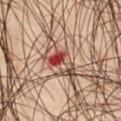Part of a total-body skin-imaging series; this lesion was reviewed on a skin check and was not flagged for biopsy. This image is a 15 mm lesion crop taken from a total-body photograph. From the right thigh. This is a cross-polarized tile. An algorithmic analysis of the crop reported an automated nevus-likeness rating near 0 out of 100 and a detector confidence of about 100 out of 100 that the crop contains a lesion. A male patient, approximately 45 years of age.